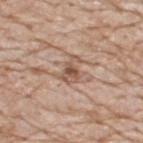{
  "biopsy_status": "not biopsied; imaged during a skin examination",
  "patient": {
    "sex": "male",
    "age_approx": 80
  },
  "site": "upper back",
  "image": {
    "source": "total-body photography crop",
    "field_of_view_mm": 15
  },
  "automated_metrics": {
    "area_mm2_approx": 5.0,
    "eccentricity": 0.7,
    "shape_asymmetry": 0.4,
    "cielab_L": 54,
    "cielab_a": 19,
    "cielab_b": 28,
    "vs_skin_darker_L": 11.0,
    "vs_skin_contrast_norm": 7.5
  }
}